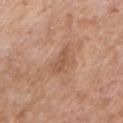Background:
Automated image analysis of the tile measured a lesion area of about 3.5 mm², a shape eccentricity near 0.9, and a shape-asymmetry score of about 0.4 (0 = symmetric). The analysis additionally found border irregularity of about 4.5 on a 0–10 scale, internal color variation of about 0.5 on a 0–10 scale, and peripheral color asymmetry of about 0. A close-up tile cropped from a whole-body skin photograph, about 15 mm across. On the front of the torso. The lesion's longest dimension is about 3 mm. Imaged with white-light lighting. A male subject in their 60s.